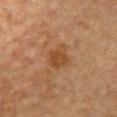No biopsy was performed on this lesion — it was imaged during a full skin examination and was not determined to be concerning.
Located on the chest.
A 15 mm crop from a total-body photograph taken for skin-cancer surveillance.
A female subject aged 48–52.
The lesion-visualizer software estimated a border-irregularity rating of about 2.5/10, internal color variation of about 2.5 on a 0–10 scale, and a peripheral color-asymmetry measure near 1. The software also gave an automated nevus-likeness rating near 35 out of 100.
About 3 mm across.
Imaged with cross-polarized lighting.